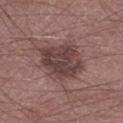  biopsy_status: not biopsied; imaged during a skin examination
  lesion_size:
    long_diameter_mm_approx: 5.5
  automated_metrics:
    area_mm2_approx: 20.0
    eccentricity: 0.6
    shape_asymmetry: 0.15
    cielab_L: 41
    cielab_a: 18
    cielab_b: 19
    vs_skin_darker_L: 11.0
    vs_skin_contrast_norm: 8.5
    border_irregularity_0_10: 2.0
    color_variation_0_10: 4.0
    nevus_likeness_0_100: 30
    lesion_detection_confidence_0_100: 100
  patient:
    sex: male
    age_approx: 60
  lighting: white-light
  image:
    source: total-body photography crop
    field_of_view_mm: 15
  site: right lower leg